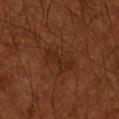The lesion was tiled from a total-body skin photograph and was not biopsied. The lesion is located on the left arm. A male patient aged 48–52. Automated tile analysis of the lesion measured a footprint of about 10 mm², a shape eccentricity near 0.75, and two-axis asymmetry of about 0.3. It also reported an average lesion color of about L≈27 a*≈21 b*≈29 (CIELAB) and a lesion–skin lightness drop of about 5. The software also gave border irregularity of about 3.5 on a 0–10 scale, internal color variation of about 2.5 on a 0–10 scale, and radial color variation of about 1. It also reported a classifier nevus-likeness of about 0/100. A 15 mm crop from a total-body photograph taken for skin-cancer surveillance. Measured at roughly 4.5 mm in maximum diameter.